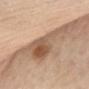Clinical impression:
This lesion was catalogued during total-body skin photography and was not selected for biopsy.
Acquisition and patient details:
A 15 mm close-up tile from a total-body photography series done for melanoma screening. From the chest. The patient is a female in their mid- to late 60s.